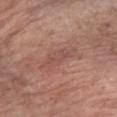Imaged during a routine full-body skin examination; the lesion was not biopsied and no histopathology is available. Automated image analysis of the tile measured a lesion area of about 7.5 mm², a shape eccentricity near 0.95, and two-axis asymmetry of about 0.3. The software also gave a lesion color around L≈51 a*≈23 b*≈24 in CIELAB, a lesion–skin lightness drop of about 7, and a normalized lesion–skin contrast near 5. The subject is a male in their 60s. Located on the left forearm. About 5 mm across. Captured under white-light illumination. A lesion tile, about 15 mm wide, cut from a 3D total-body photograph.